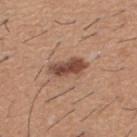Imaged during a routine full-body skin examination; the lesion was not biopsied and no histopathology is available.
A 15 mm close-up tile from a total-body photography series done for melanoma screening.
A male patient about 60 years old.
The lesion is on the upper back.